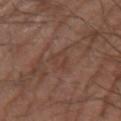A male subject, about 65 years old. A 15 mm close-up tile from a total-body photography series done for melanoma screening. Located on the left forearm.